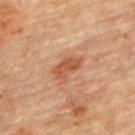  biopsy_status: not biopsied; imaged during a skin examination
  image:
    source: total-body photography crop
    field_of_view_mm: 15
  site: back
  patient:
    sex: male
    age_approx: 85+
  lesion_size:
    long_diameter_mm_approx: 3.5
  lighting: cross-polarized